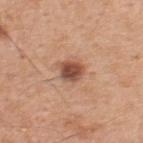biopsy status = no biopsy performed (imaged during a skin exam) | anatomic site = the upper back | tile lighting = white-light illumination | lesion size = ~3 mm (longest diameter) | imaging modality = total-body-photography crop, ~15 mm field of view | subject = male, aged 48–52.